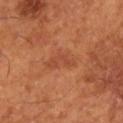A close-up tile cropped from a whole-body skin photograph, about 15 mm across. Automated image analysis of the tile measured a border-irregularity rating of about 4.5/10. A male subject roughly 65 years of age. About 4 mm across. Imaged with cross-polarized lighting. From the leg.